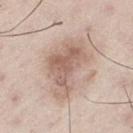Case summary:
- notes — no biopsy performed (imaged during a skin exam)
- subject — male, aged 43–47
- lesion size — about 7 mm
- tile lighting — white-light
- site — the left thigh
- acquisition — ~15 mm tile from a whole-body skin photo